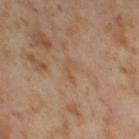The lesion was photographed on a routine skin check and not biopsied; there is no pathology result.
A female patient, aged 53 to 57.
Cropped from a total-body skin-imaging series; the visible field is about 15 mm.
This is a cross-polarized tile.
Located on the right thigh.
The lesion-visualizer software estimated an average lesion color of about L≈54 a*≈17 b*≈33 (CIELAB) and about 5 CIELAB-L* units darker than the surrounding skin. And it measured a nevus-likeness score of about 0/100 and a lesion-detection confidence of about 95/100.
Approximately 2.5 mm at its widest.